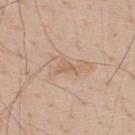Imaged during a routine full-body skin examination; the lesion was not biopsied and no histopathology is available. Cropped from a total-body skin-imaging series; the visible field is about 15 mm. The recorded lesion diameter is about 3.5 mm. A male subject aged 38–42. Located on the upper back.